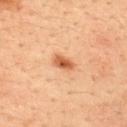Assessment: No biopsy was performed on this lesion — it was imaged during a full skin examination and was not determined to be concerning. Context: Automated image analysis of the tile measured a lesion area of about 3.5 mm², an eccentricity of roughly 0.8, and a symmetry-axis asymmetry near 0.2. It also reported a mean CIELAB color near L≈55 a*≈26 b*≈38 and a lesion-to-skin contrast of about 9 (normalized; higher = more distinct). It also reported a classifier nevus-likeness of about 100/100 and a lesion-detection confidence of about 100/100. The patient is a male aged 33 to 37. The lesion is located on the upper back. This is a cross-polarized tile. A close-up tile cropped from a whole-body skin photograph, about 15 mm across. Measured at roughly 2.5 mm in maximum diameter.